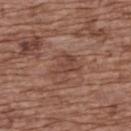Assessment: This lesion was catalogued during total-body skin photography and was not selected for biopsy. Context: This image is a 15 mm lesion crop taken from a total-body photograph. A female patient, about 75 years old. An algorithmic analysis of the crop reported a border-irregularity index near 5.5/10 and peripheral color asymmetry of about 1. And it measured a nevus-likeness score of about 0/100. On the back.